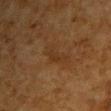Part of a total-body skin-imaging series; this lesion was reviewed on a skin check and was not flagged for biopsy. Cropped from a total-body skin-imaging series; the visible field is about 15 mm. The lesion is located on the front of the torso. A male patient, aged 58 to 62.